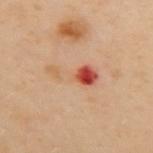Case summary:
• notes: no biopsy performed (imaged during a skin exam)
• patient: female, aged 58 to 62
• site: the back
• image: 15 mm crop, total-body photography